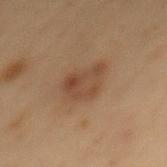This is a cross-polarized tile.
Approximately 4.5 mm at its widest.
An algorithmic analysis of the crop reported a lesion area of about 11 mm², an eccentricity of roughly 0.6, and a shape-asymmetry score of about 0.3 (0 = symmetric). The software also gave a mean CIELAB color near L≈37 a*≈16 b*≈26, roughly 7 lightness units darker than nearby skin, and a lesion-to-skin contrast of about 6 (normalized; higher = more distinct).
The lesion is located on the back.
A 15 mm crop from a total-body photograph taken for skin-cancer surveillance.
The subject is a male roughly 55 years of age.